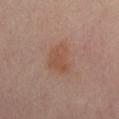Q: Was this lesion biopsied?
A: catalogued during a skin exam; not biopsied
Q: Illumination type?
A: cross-polarized illumination
Q: Lesion size?
A: ≈4 mm
Q: Lesion location?
A: the abdomen
Q: Patient demographics?
A: male, in their mid-60s
Q: How was this image acquired?
A: total-body-photography crop, ~15 mm field of view